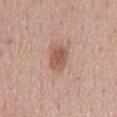Impression:
The lesion was tiled from a total-body skin photograph and was not biopsied.
Background:
From the mid back. The patient is a female about 65 years old. A 15 mm crop from a total-body photograph taken for skin-cancer surveillance.